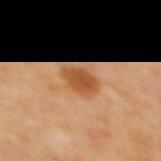notes: catalogued during a skin exam; not biopsied
automated lesion analysis: an average lesion color of about L≈52 a*≈24 b*≈40 (CIELAB); border irregularity of about 1.5 on a 0–10 scale and internal color variation of about 3 on a 0–10 scale; a classifier nevus-likeness of about 95/100 and lesion-presence confidence of about 100/100
lighting: cross-polarized illumination
imaging modality: ~15 mm tile from a whole-body skin photo
lesion size: ~3.5 mm (longest diameter)
anatomic site: the mid back
patient: male, in their mid-60s